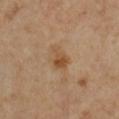Imaged during a routine full-body skin examination; the lesion was not biopsied and no histopathology is available.
A roughly 15 mm field-of-view crop from a total-body skin photograph.
On the right lower leg.
Captured under cross-polarized illumination.
Longest diameter approximately 3 mm.
The subject is a female aged around 50.